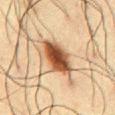follow-up = catalogued during a skin exam; not biopsied | site = the abdomen | lesion diameter = ≈5 mm | image source = 15 mm crop, total-body photography | subject = male, in their 60s.